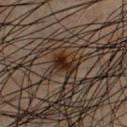| feature | finding |
|---|---|
| workup | catalogued during a skin exam; not biopsied |
| patient | male, aged around 50 |
| site | the chest |
| imaging modality | ~15 mm crop, total-body skin-cancer survey |